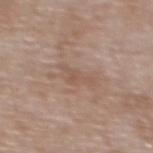| key | value |
|---|---|
| workup | catalogued during a skin exam; not biopsied |
| size | ≈3.5 mm |
| subject | male, aged approximately 50 |
| imaging modality | ~15 mm crop, total-body skin-cancer survey |
| location | the back |
| image-analysis metrics | an area of roughly 4 mm², a shape eccentricity near 0.85, and two-axis asymmetry of about 0.55; about 6 CIELAB-L* units darker than the surrounding skin and a normalized border contrast of about 4.5; radial color variation of about 0.5; a classifier nevus-likeness of about 0/100 and a detector confidence of about 100 out of 100 that the crop contains a lesion |